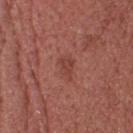This lesion was catalogued during total-body skin photography and was not selected for biopsy. The tile uses white-light illumination. Cropped from a total-body skin-imaging series; the visible field is about 15 mm. A female patient, in their mid- to late 60s. From the head or neck. Automated tile analysis of the lesion measured two-axis asymmetry of about 0.3. The analysis additionally found a classifier nevus-likeness of about 0/100. Approximately 2.5 mm at its widest.